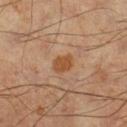The lesion was photographed on a routine skin check and not biopsied; there is no pathology result. On the left lower leg. A male subject aged 58 to 62. A close-up tile cropped from a whole-body skin photograph, about 15 mm across.